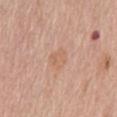Q: Was this lesion biopsied?
A: imaged on a skin check; not biopsied
Q: What are the patient's age and sex?
A: male, about 65 years old
Q: Lesion size?
A: about 3 mm
Q: How was this image acquired?
A: ~15 mm tile from a whole-body skin photo
Q: Where on the body is the lesion?
A: the back